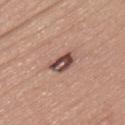Clinical impression: Recorded during total-body skin imaging; not selected for excision or biopsy. Clinical summary: Measured at roughly 3.5 mm in maximum diameter. Imaged with white-light lighting. The patient is a female about 55 years old. Cropped from a total-body skin-imaging series; the visible field is about 15 mm. An algorithmic analysis of the crop reported an area of roughly 5.5 mm² and a shape-asymmetry score of about 0.25 (0 = symmetric). The analysis additionally found border irregularity of about 2.5 on a 0–10 scale, a color-variation rating of about 8.5/10, and a peripheral color-asymmetry measure near 4. Located on the left thigh.